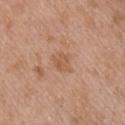A 15 mm close-up extracted from a 3D total-body photography capture. A male patient in their mid- to late 50s. Located on the right upper arm.